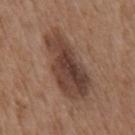• biopsy status · total-body-photography surveillance lesion; no biopsy
• location · the chest
• image-analysis metrics · a footprint of about 25 mm² and a shape-asymmetry score of about 0.25 (0 = symmetric); a mean CIELAB color near L≈41 a*≈18 b*≈25 and a normalized lesion–skin contrast near 10
• patient · male, in their mid-70s
• imaging modality · total-body-photography crop, ~15 mm field of view
• illumination · white-light